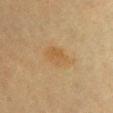Part of a total-body skin-imaging series; this lesion was reviewed on a skin check and was not flagged for biopsy.